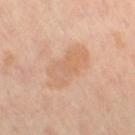This is a cross-polarized tile.
The recorded lesion diameter is about 6 mm.
On the right thigh.
The subject is a female aged around 50.
A lesion tile, about 15 mm wide, cut from a 3D total-body photograph.
The lesion-visualizer software estimated a footprint of about 16 mm² and a symmetry-axis asymmetry near 0.15. The analysis additionally found an average lesion color of about L≈66 a*≈21 b*≈34 (CIELAB), roughly 7 lightness units darker than nearby skin, and a lesion-to-skin contrast of about 5 (normalized; higher = more distinct). And it measured a border-irregularity rating of about 2.5/10, a color-variation rating of about 4/10, and peripheral color asymmetry of about 1.5.